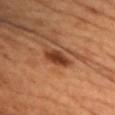<record>
  <biopsy_status>not biopsied; imaged during a skin examination</biopsy_status>
  <image>
    <source>total-body photography crop</source>
    <field_of_view_mm>15</field_of_view_mm>
  </image>
  <automated_metrics>
    <area_mm2_approx>6.0</area_mm2_approx>
    <eccentricity>0.85</eccentricity>
    <cielab_L>41</cielab_L>
    <cielab_a>26</cielab_a>
    <cielab_b>34</cielab_b>
    <vs_skin_contrast_norm>10.0</vs_skin_contrast_norm>
    <border_irregularity_0_10>2.5</border_irregularity_0_10>
    <color_variation_0_10>3.0</color_variation_0_10>
    <nevus_likeness_0_100>95</nevus_likeness_0_100>
  </automated_metrics>
  <lighting>cross-polarized</lighting>
  <patient>
    <sex>male</sex>
    <age_approx>40</age_approx>
  </patient>
  <lesion_size>
    <long_diameter_mm_approx>3.5</long_diameter_mm_approx>
  </lesion_size>
  <site>chest</site>
</record>